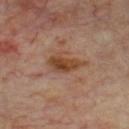<tbp_lesion>
<biopsy_status>not biopsied; imaged during a skin examination</biopsy_status>
<lighting>cross-polarized</lighting>
<image>
  <source>total-body photography crop</source>
  <field_of_view_mm>15</field_of_view_mm>
</image>
<site>chest</site>
<automated_metrics>
  <cielab_L>41</cielab_L>
  <cielab_a>20</cielab_a>
  <cielab_b>31</cielab_b>
  <vs_skin_darker_L>10.0</vs_skin_darker_L>
  <vs_skin_contrast_norm>9.5</vs_skin_contrast_norm>
  <nevus_likeness_0_100>5</nevus_likeness_0_100>
  <lesion_detection_confidence_0_100>100</lesion_detection_confidence_0_100>
</automated_metrics>
<patient>
  <sex>male</sex>
  <age_approx>70</age_approx>
</patient>
</tbp_lesion>